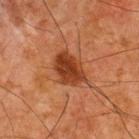Acquisition and patient details:
A 15 mm close-up extracted from a 3D total-body photography capture. A male subject, approximately 65 years of age. About 4.5 mm across. From the upper back. Captured under cross-polarized illumination.